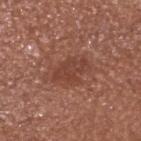anatomic site: the head or neck
subject: male, approximately 65 years of age
size: about 4.5 mm
image-analysis metrics: a lesion area of about 8 mm² and two-axis asymmetry of about 0.25; a lesion color around L≈42 a*≈24 b*≈28 in CIELAB, about 8 CIELAB-L* units darker than the surrounding skin, and a lesion-to-skin contrast of about 6 (normalized; higher = more distinct); a border-irregularity index near 3.5/10 and a color-variation rating of about 2.5/10; a classifier nevus-likeness of about 15/100 and a detector confidence of about 100 out of 100 that the crop contains a lesion
acquisition: ~15 mm crop, total-body skin-cancer survey